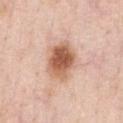Recorded during total-body skin imaging; not selected for excision or biopsy.
The subject is a male in their 50s.
A 15 mm close-up tile from a total-body photography series done for melanoma screening.
Captured under white-light illumination.
The lesion's longest dimension is about 5 mm.
The lesion is on the chest.